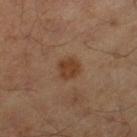- follow-up — total-body-photography surveillance lesion; no biopsy
- illumination — cross-polarized
- patient — male, in their 60s
- location — the left thigh
- diameter — ≈2.5 mm
- image source — 15 mm crop, total-body photography
- TBP lesion metrics — a symmetry-axis asymmetry near 0.15; a border-irregularity index near 1.5/10 and a within-lesion color-variation index near 1.5/10